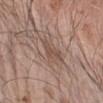The lesion was photographed on a routine skin check and not biopsied; there is no pathology result. Measured at roughly 2.5 mm in maximum diameter. Automated tile analysis of the lesion measured a mean CIELAB color near L≈49 a*≈17 b*≈24 and a lesion–skin lightness drop of about 7. The analysis additionally found a border-irregularity index near 5.5/10 and radial color variation of about 0. It also reported a classifier nevus-likeness of about 0/100 and a lesion-detection confidence of about 95/100. Imaged with white-light lighting. A male subject aged around 70. Cropped from a total-body skin-imaging series; the visible field is about 15 mm. On the left forearm.